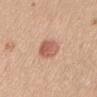Impression: The lesion was tiled from a total-body skin photograph and was not biopsied. Background: About 4 mm across. The lesion is on the head or neck. This image is a 15 mm lesion crop taken from a total-body photograph. A female subject, aged 38–42. Captured under white-light illumination.